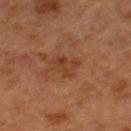* diameter — about 3 mm
* image-analysis metrics — a lesion area of about 6 mm² and an eccentricity of roughly 0.5; a lesion color around L≈39 a*≈23 b*≈32 in CIELAB, a lesion–skin lightness drop of about 6, and a normalized border contrast of about 5.5; a within-lesion color-variation index near 3.5/10; an automated nevus-likeness rating near 0 out of 100 and lesion-presence confidence of about 100/100
* acquisition — 15 mm crop, total-body photography
* patient — female, aged around 65
* illumination — cross-polarized illumination
* anatomic site — the right lower leg